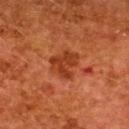Notes:
* follow-up: imaged on a skin check; not biopsied
* subject: female, aged 48 to 52
* lesion diameter: ~3.5 mm (longest diameter)
* body site: the upper back
* image: total-body-photography crop, ~15 mm field of view
* automated lesion analysis: an area of roughly 9 mm² and a shape eccentricity near 0.45; a classifier nevus-likeness of about 0/100 and lesion-presence confidence of about 100/100
* tile lighting: cross-polarized illumination